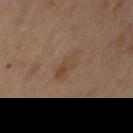Clinical impression:
Part of a total-body skin-imaging series; this lesion was reviewed on a skin check and was not flagged for biopsy.
Acquisition and patient details:
The total-body-photography lesion software estimated a lesion-detection confidence of about 100/100. A lesion tile, about 15 mm wide, cut from a 3D total-body photograph. The subject is a female in their mid-50s. The lesion is located on the left upper arm. Approximately 3 mm at its widest. The tile uses cross-polarized illumination.